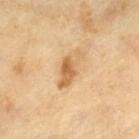Recorded during total-body skin imaging; not selected for excision or biopsy. A male subject aged 63 to 67. Cropped from a whole-body photographic skin survey; the tile spans about 15 mm. The lesion-visualizer software estimated an outline eccentricity of about 0.9 (0 = round, 1 = elongated) and a symmetry-axis asymmetry near 0.3. The software also gave a lesion color around L≈62 a*≈19 b*≈41 in CIELAB. It also reported a border-irregularity rating of about 4.5/10, a within-lesion color-variation index near 3/10, and a peripheral color-asymmetry measure near 0.5. It also reported an automated nevus-likeness rating near 40 out of 100 and a detector confidence of about 100 out of 100 that the crop contains a lesion.